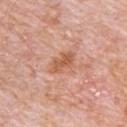notes = total-body-photography surveillance lesion; no biopsy | image source = 15 mm crop, total-body photography | lesion size = ~3.5 mm (longest diameter) | illumination = white-light | anatomic site = the chest | patient = male, aged around 80 | image-analysis metrics = a border-irregularity rating of about 2.5/10 and a color-variation rating of about 3.5/10.